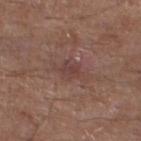Assessment: Imaged during a routine full-body skin examination; the lesion was not biopsied and no histopathology is available. Acquisition and patient details: Imaged with white-light lighting. On the leg. The subject is a male aged 78–82. An algorithmic analysis of the crop reported a shape eccentricity near 0.7 and a symmetry-axis asymmetry near 0.3. This image is a 15 mm lesion crop taken from a total-body photograph. Approximately 3 mm at its widest.